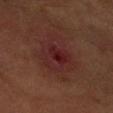The total-body-photography lesion software estimated a footprint of about 16 mm² and an outline eccentricity of about 0.65 (0 = round, 1 = elongated). It also reported a border-irregularity rating of about 4/10, a within-lesion color-variation index near 5/10, and a peripheral color-asymmetry measure near 1.5. The software also gave an automated nevus-likeness rating near 0 out of 100 and lesion-presence confidence of about 100/100. Cropped from a total-body skin-imaging series; the visible field is about 15 mm. This is a cross-polarized tile. A male subject, aged approximately 60. Measured at roughly 5.5 mm in maximum diameter. Located on the right forearm.